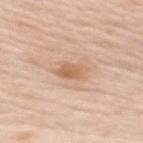Assessment: The lesion was photographed on a routine skin check and not biopsied; there is no pathology result.